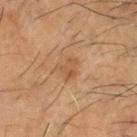Part of a total-body skin-imaging series; this lesion was reviewed on a skin check and was not flagged for biopsy. A 15 mm close-up extracted from a 3D total-body photography capture. A male patient, aged around 60. The lesion's longest dimension is about 3 mm.